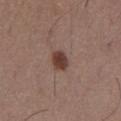Part of a total-body skin-imaging series; this lesion was reviewed on a skin check and was not flagged for biopsy. This image is a 15 mm lesion crop taken from a total-body photograph. Longest diameter approximately 2.5 mm. On the chest. The tile uses white-light illumination. A male patient in their 40s.